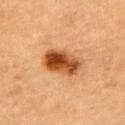follow-up = imaged on a skin check; not biopsied | site = the chest | subject = male, aged approximately 85 or older | lesion size = about 5 mm | automated lesion analysis = a footprint of about 13 mm², an eccentricity of roughly 0.8, and a symmetry-axis asymmetry near 0.15 | illumination = cross-polarized illumination | acquisition = 15 mm crop, total-body photography.